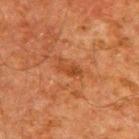Assessment:
This lesion was catalogued during total-body skin photography and was not selected for biopsy.
Image and clinical context:
The tile uses cross-polarized illumination. A male patient aged 58–62. A roughly 15 mm field-of-view crop from a total-body skin photograph. Located on the upper back.